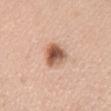Q: Was this lesion biopsied?
A: imaged on a skin check; not biopsied
Q: What did automated image analysis measure?
A: a mean CIELAB color near L≈57 a*≈22 b*≈30, about 16 CIELAB-L* units darker than the surrounding skin, and a normalized border contrast of about 10.5
Q: Who is the patient?
A: female, approximately 40 years of age
Q: Illumination type?
A: white-light
Q: What is the imaging modality?
A: ~15 mm crop, total-body skin-cancer survey
Q: What is the anatomic site?
A: the chest
Q: Lesion size?
A: ~3.5 mm (longest diameter)